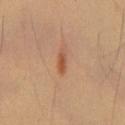{
  "patient": {
    "sex": "male",
    "age_approx": 55
  },
  "site": "chest",
  "lesion_size": {
    "long_diameter_mm_approx": 3.0
  },
  "image": {
    "source": "total-body photography crop",
    "field_of_view_mm": 15
  },
  "automated_metrics": {
    "area_mm2_approx": 3.0,
    "eccentricity": 0.9,
    "cielab_L": 50,
    "cielab_a": 24,
    "cielab_b": 33,
    "vs_skin_darker_L": 9.0,
    "vs_skin_contrast_norm": 7.0
  }
}